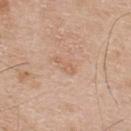| field | value |
|---|---|
| notes | total-body-photography surveillance lesion; no biopsy |
| location | the upper back |
| lighting | white-light |
| subject | male, in their mid-50s |
| image source | 15 mm crop, total-body photography |
| lesion size | ≈3 mm |